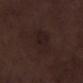Notes:
– workup — imaged on a skin check; not biopsied
– site — the right lower leg
– patient — male, aged approximately 70
– imaging modality — ~15 mm tile from a whole-body skin photo
– size — ~4 mm (longest diameter)
– tile lighting — white-light illumination